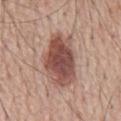{
  "biopsy_status": "not biopsied; imaged during a skin examination",
  "site": "mid back",
  "image": {
    "source": "total-body photography crop",
    "field_of_view_mm": 15
  },
  "automated_metrics": {
    "area_mm2_approx": 21.0,
    "eccentricity": 0.85,
    "shape_asymmetry": 0.15,
    "cielab_L": 49,
    "cielab_a": 22,
    "cielab_b": 24,
    "vs_skin_darker_L": 15.0,
    "border_irregularity_0_10": 2.0,
    "peripheral_color_asymmetry": 1.5,
    "lesion_detection_confidence_0_100": 100
  },
  "patient": {
    "sex": "male",
    "age_approx": 65
  },
  "lesion_size": {
    "long_diameter_mm_approx": 7.0
  }
}